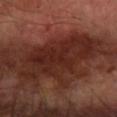Captured during whole-body skin photography for melanoma surveillance; the lesion was not biopsied. Longest diameter approximately 10 mm. Cropped from a whole-body photographic skin survey; the tile spans about 15 mm. The lesion-visualizer software estimated a classifier nevus-likeness of about 0/100 and lesion-presence confidence of about 85/100. A male patient approximately 65 years of age. On the right forearm.